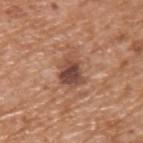| key | value |
|---|---|
| body site | the upper back |
| lesion diameter | ≈4 mm |
| tile lighting | white-light |
| image-analysis metrics | an area of roughly 8.5 mm² and a symmetry-axis asymmetry near 0.3; a nevus-likeness score of about 10/100 and lesion-presence confidence of about 100/100 |
| image source | ~15 mm tile from a whole-body skin photo |
| patient | male, aged approximately 65 |